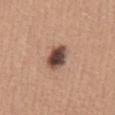Impression:
Recorded during total-body skin imaging; not selected for excision or biopsy.
Acquisition and patient details:
An algorithmic analysis of the crop reported a footprint of about 7.5 mm². And it measured a lesion–skin lightness drop of about 19 and a normalized lesion–skin contrast near 13. It also reported a border-irregularity index near 1.5/10 and peripheral color asymmetry of about 2. Cropped from a whole-body photographic skin survey; the tile spans about 15 mm. This is a white-light tile. A female patient, about 40 years old. The lesion is located on the abdomen.